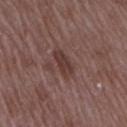Imaged during a routine full-body skin examination; the lesion was not biopsied and no histopathology is available.
A lesion tile, about 15 mm wide, cut from a 3D total-body photograph.
About 4 mm across.
The lesion is located on the right upper arm.
A female subject, in their 70s.
This is a white-light tile.